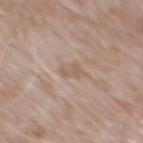biopsy status=imaged on a skin check; not biopsied
image=15 mm crop, total-body photography
automated metrics=a mean CIELAB color near L≈58 a*≈16 b*≈26, a lesion–skin lightness drop of about 7, and a normalized lesion–skin contrast near 5.5; a border-irregularity index near 3/10, internal color variation of about 1 on a 0–10 scale, and a peripheral color-asymmetry measure near 0
subject=male, roughly 50 years of age
lighting=white-light
anatomic site=the upper back